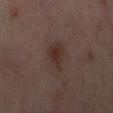Q: Is there a histopathology result?
A: no biopsy performed (imaged during a skin exam)